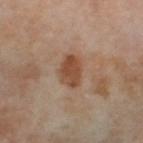  biopsy_status: not biopsied; imaged during a skin examination
  patient:
    sex: female
    age_approx: 55
  automated_metrics:
    cielab_L: 48
    cielab_a: 20
    cielab_b: 31
    vs_skin_darker_L: 11.0
    vs_skin_contrast_norm: 9.0
    border_irregularity_0_10: 2.0
    color_variation_0_10: 3.0
    nevus_likeness_0_100: 40
    lesion_detection_confidence_0_100: 100
  site: left thigh
  lighting: cross-polarized
  lesion_size:
    long_diameter_mm_approx: 3.5
  image:
    source: total-body photography crop
    field_of_view_mm: 15